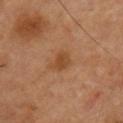{
  "lighting": "cross-polarized",
  "site": "front of the torso",
  "image": {
    "source": "total-body photography crop",
    "field_of_view_mm": 15
  },
  "patient": {
    "sex": "male",
    "age_approx": 55
  }
}